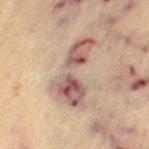Impression: The lesion was tiled from a total-body skin photograph and was not biopsied. Context: Approximately 7.5 mm at its widest. The lesion-visualizer software estimated a footprint of about 19 mm², an eccentricity of roughly 0.9, and a shape-asymmetry score of about 0.45 (0 = symmetric). And it measured a lesion–skin lightness drop of about 13 and a normalized border contrast of about 9.5. The software also gave border irregularity of about 7 on a 0–10 scale. It also reported a nevus-likeness score of about 0/100 and a detector confidence of about 50 out of 100 that the crop contains a lesion. Cropped from a total-body skin-imaging series; the visible field is about 15 mm. On the left thigh. Captured under cross-polarized illumination. A female patient aged around 65.